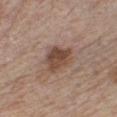No biopsy was performed on this lesion — it was imaged during a full skin examination and was not determined to be concerning. The lesion-visualizer software estimated a lesion area of about 8.5 mm², a shape eccentricity near 0.55, and two-axis asymmetry of about 0.3. And it measured a border-irregularity index near 3/10, a color-variation rating of about 4/10, and a peripheral color-asymmetry measure near 1.5. The analysis additionally found a nevus-likeness score of about 70/100 and lesion-presence confidence of about 100/100. The recorded lesion diameter is about 4 mm. A 15 mm close-up tile from a total-body photography series done for melanoma screening. A male patient, aged approximately 60. The lesion is on the chest.